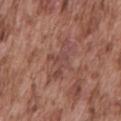No biopsy was performed on this lesion — it was imaged during a full skin examination and was not determined to be concerning. Automated image analysis of the tile measured a footprint of about 7.5 mm², an outline eccentricity of about 0.85 (0 = round, 1 = elongated), and a symmetry-axis asymmetry near 0.4. The analysis additionally found an average lesion color of about L≈45 a*≈22 b*≈24 (CIELAB) and a normalized border contrast of about 5.5. And it measured a classifier nevus-likeness of about 0/100 and a detector confidence of about 70 out of 100 that the crop contains a lesion. The lesion's longest dimension is about 5 mm. A male patient aged 73–77. This is a white-light tile. A roughly 15 mm field-of-view crop from a total-body skin photograph. On the mid back.